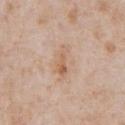The lesion was tiled from a total-body skin photograph and was not biopsied.
On the front of the torso.
This image is a 15 mm lesion crop taken from a total-body photograph.
Measured at roughly 4 mm in maximum diameter.
An algorithmic analysis of the crop reported a mean CIELAB color near L≈62 a*≈18 b*≈32 and a lesion–skin lightness drop of about 8. The analysis additionally found a border-irregularity rating of about 4.5/10 and a peripheral color-asymmetry measure near 1. The analysis additionally found a lesion-detection confidence of about 100/100.
The tile uses white-light illumination.
A male subject, in their mid- to late 60s.